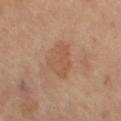Recorded during total-body skin imaging; not selected for excision or biopsy. The lesion is located on the leg. Longest diameter approximately 4.5 mm. A roughly 15 mm field-of-view crop from a total-body skin photograph. A female subject, aged 63–67.